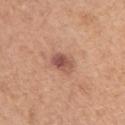Captured during whole-body skin photography for melanoma surveillance; the lesion was not biopsied.
A 15 mm close-up tile from a total-body photography series done for melanoma screening.
From the left upper arm.
A female patient, about 65 years old.